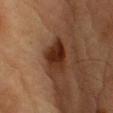Recorded during total-body skin imaging; not selected for excision or biopsy.
A roughly 15 mm field-of-view crop from a total-body skin photograph.
A male patient aged around 85 or older.
Automated tile analysis of the lesion measured a border-irregularity index near 2.5/10, a color-variation rating of about 4.5/10, and peripheral color asymmetry of about 1.5.
From the right upper arm.
This is a cross-polarized tile.
Measured at roughly 4 mm in maximum diameter.